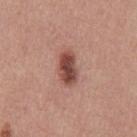Q: How was this image acquired?
A: 15 mm crop, total-body photography
Q: Lesion size?
A: about 4 mm
Q: What are the patient's age and sex?
A: male, aged 38–42
Q: What is the anatomic site?
A: the chest